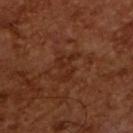A lesion tile, about 15 mm wide, cut from a 3D total-body photograph.
A male subject, aged around 65.